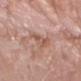Captured during whole-body skin photography for melanoma surveillance; the lesion was not biopsied.